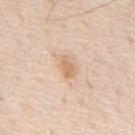Assessment: The lesion was photographed on a routine skin check and not biopsied; there is no pathology result. Background: The patient is a male in their 80s. A roughly 15 mm field-of-view crop from a total-body skin photograph. Approximately 2.5 mm at its widest. The lesion is located on the mid back.